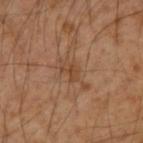Impression: This lesion was catalogued during total-body skin photography and was not selected for biopsy. Acquisition and patient details: From the back. A 15 mm close-up tile from a total-body photography series done for melanoma screening. The patient is a male aged 53–57. Imaged with cross-polarized lighting. Approximately 2.5 mm at its widest.